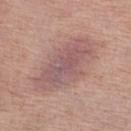<lesion>
<biopsy_status>not biopsied; imaged during a skin examination</biopsy_status>
<image>
  <source>total-body photography crop</source>
  <field_of_view_mm>15</field_of_view_mm>
</image>
<site>left lower leg</site>
<lighting>white-light</lighting>
<patient>
  <sex>female</sex>
  <age_approx>75</age_approx>
</patient>
<lesion_size>
  <long_diameter_mm_approx>8.5</long_diameter_mm_approx>
</lesion_size>
</lesion>